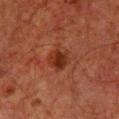follow-up = imaged on a skin check; not biopsied | imaging modality = 15 mm crop, total-body photography | subject = male, in their 60s | site = the chest.